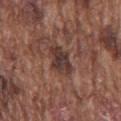<case>
  <biopsy_status>not biopsied; imaged during a skin examination</biopsy_status>
  <patient>
    <sex>male</sex>
    <age_approx>75</age_approx>
  </patient>
  <image>
    <source>total-body photography crop</source>
    <field_of_view_mm>15</field_of_view_mm>
  </image>
  <site>chest</site>
  <lesion_size>
    <long_diameter_mm_approx>4.0</long_diameter_mm_approx>
  </lesion_size>
  <lighting>white-light</lighting>
</case>